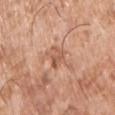workup = imaged on a skin check; not biopsied
anatomic site = the chest
diameter = ≈2.5 mm
subject = male, approximately 75 years of age
lighting = white-light illumination
image = ~15 mm tile from a whole-body skin photo